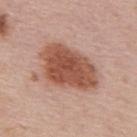<lesion>
<biopsy_status>not biopsied; imaged during a skin examination</biopsy_status>
<patient>
  <sex>male</sex>
  <age_approx>55</age_approx>
</patient>
<image>
  <source>total-body photography crop</source>
  <field_of_view_mm>15</field_of_view_mm>
</image>
<site>back</site>
<lesion_size>
  <long_diameter_mm_approx>7.5</long_diameter_mm_approx>
</lesion_size>
<lighting>white-light</lighting>
</lesion>